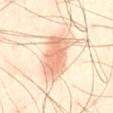<tbp_lesion>
  <biopsy_status>not biopsied; imaged during a skin examination</biopsy_status>
  <lighting>cross-polarized</lighting>
  <site>front of the torso</site>
  <lesion_size>
    <long_diameter_mm_approx>6.5</long_diameter_mm_approx>
  </lesion_size>
  <image>
    <source>total-body photography crop</source>
    <field_of_view_mm>15</field_of_view_mm>
  </image>
  <patient>
    <sex>male</sex>
    <age_approx>40</age_approx>
  </patient>
</tbp_lesion>